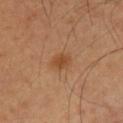Background: The patient is a male about 30 years old. The recorded lesion diameter is about 2.5 mm. On the chest. A 15 mm close-up tile from a total-body photography series done for melanoma screening.